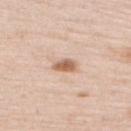Findings:
– biopsy status · imaged on a skin check; not biopsied
– site · the upper back
– acquisition · total-body-photography crop, ~15 mm field of view
– automated lesion analysis · a shape eccentricity near 0.85; an average lesion color of about L≈63 a*≈20 b*≈31 (CIELAB), roughly 13 lightness units darker than nearby skin, and a normalized border contrast of about 8.5; an automated nevus-likeness rating near 95 out of 100 and lesion-presence confidence of about 100/100
– subject · female, about 65 years old